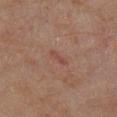Q: Was this lesion biopsied?
A: no biopsy performed (imaged during a skin exam)